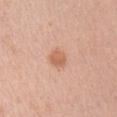Findings:
– follow-up: no biopsy performed (imaged during a skin exam)
– site: the right upper arm
– automated lesion analysis: border irregularity of about 2 on a 0–10 scale, internal color variation of about 2 on a 0–10 scale, and a peripheral color-asymmetry measure near 1; an automated nevus-likeness rating near 80 out of 100 and a lesion-detection confidence of about 100/100
– size: ≈2.5 mm
– subject: female, aged approximately 45
– image: 15 mm crop, total-body photography
– tile lighting: white-light